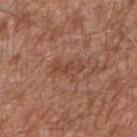notes: imaged on a skin check; not biopsied | patient: male, aged around 55 | diameter: ~3.5 mm (longest diameter) | automated lesion analysis: an eccentricity of roughly 0.8 and a symmetry-axis asymmetry near 0.4; a border-irregularity rating of about 5/10, internal color variation of about 2.5 on a 0–10 scale, and a peripheral color-asymmetry measure near 1 | lighting: white-light illumination | location: the right forearm | imaging modality: total-body-photography crop, ~15 mm field of view.